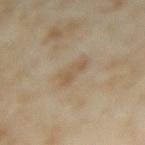Clinical summary: This is a cross-polarized tile. From the right forearm. The recorded lesion diameter is about 3 mm. Cropped from a whole-body photographic skin survey; the tile spans about 15 mm. The patient is a female about 35 years old. The lesion-visualizer software estimated a footprint of about 4 mm², an eccentricity of roughly 0.85, and a shape-asymmetry score of about 0.4 (0 = symmetric). It also reported a lesion color around L≈52 a*≈12 b*≈30 in CIELAB, roughly 7 lightness units darker than nearby skin, and a lesion-to-skin contrast of about 5.5 (normalized; higher = more distinct). The software also gave border irregularity of about 4 on a 0–10 scale, a color-variation rating of about 1.5/10, and a peripheral color-asymmetry measure near 0.5. The analysis additionally found lesion-presence confidence of about 100/100.